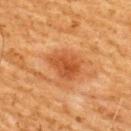Assessment: Recorded during total-body skin imaging; not selected for excision or biopsy. Background: A male subject in their 60s. Cropped from a whole-body photographic skin survey; the tile spans about 15 mm. The total-body-photography lesion software estimated an area of roughly 10 mm², a shape eccentricity near 0.65, and a shape-asymmetry score of about 0.3 (0 = symmetric). And it measured a lesion color around L≈44 a*≈26 b*≈37 in CIELAB, roughly 9 lightness units darker than nearby skin, and a lesion-to-skin contrast of about 7 (normalized; higher = more distinct). The analysis additionally found a border-irregularity rating of about 3/10 and a color-variation rating of about 3.5/10. It also reported a nevus-likeness score of about 80/100 and lesion-presence confidence of about 100/100. From the back.